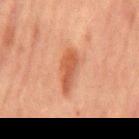Q: Was this lesion biopsied?
A: imaged on a skin check; not biopsied
Q: Where on the body is the lesion?
A: the abdomen
Q: Who is the patient?
A: male, aged around 65
Q: Automated lesion metrics?
A: a lesion area of about 8 mm², an outline eccentricity of about 0.95 (0 = round, 1 = elongated), and a shape-asymmetry score of about 0.3 (0 = symmetric); a lesion color around L≈45 a*≈24 b*≈31 in CIELAB and a normalized border contrast of about 7.5; a nevus-likeness score of about 95/100 and a detector confidence of about 100 out of 100 that the crop contains a lesion
Q: How was this image acquired?
A: ~15 mm crop, total-body skin-cancer survey
Q: What is the lesion's diameter?
A: about 5 mm